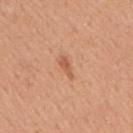Captured during whole-body skin photography for melanoma surveillance; the lesion was not biopsied.
Located on the mid back.
The recorded lesion diameter is about 3 mm.
A male subject aged 53–57.
This is a white-light tile.
An algorithmic analysis of the crop reported a lesion area of about 3 mm², a shape eccentricity near 0.9, and a symmetry-axis asymmetry near 0.4. And it measured a classifier nevus-likeness of about 10/100.
This image is a 15 mm lesion crop taken from a total-body photograph.